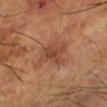{
  "biopsy_status": "not biopsied; imaged during a skin examination",
  "image": {
    "source": "total-body photography crop",
    "field_of_view_mm": 15
  },
  "patient": {
    "sex": "male",
    "age_approx": 65
  },
  "site": "left lower leg"
}